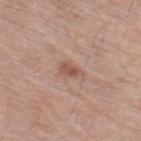Background:
A male subject in their 80s. The lesion is on the right thigh. A roughly 15 mm field-of-view crop from a total-body skin photograph. Captured under white-light illumination. The lesion's longest dimension is about 2.5 mm.